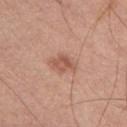follow-up=catalogued during a skin exam; not biopsied | acquisition=total-body-photography crop, ~15 mm field of view | body site=the chest | patient=male, aged 28–32.